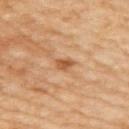follow-up=catalogued during a skin exam; not biopsied | site=the right upper arm | subject=female, aged 58–62 | image-analysis metrics=a lesion area of about 4 mm², a shape eccentricity near 0.5, and a symmetry-axis asymmetry near 0.3 | imaging modality=~15 mm crop, total-body skin-cancer survey.